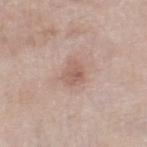notes: imaged on a skin check; not biopsied | imaging modality: total-body-photography crop, ~15 mm field of view | body site: the leg | illumination: white-light | lesion size: ≈3 mm | automated lesion analysis: an area of roughly 5.5 mm², an eccentricity of roughly 0.6, and two-axis asymmetry of about 0.35; a border-irregularity rating of about 3/10, a within-lesion color-variation index near 3/10, and a peripheral color-asymmetry measure near 1 | subject: male, roughly 80 years of age.